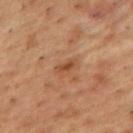No biopsy was performed on this lesion — it was imaged during a full skin examination and was not determined to be concerning.
This is a cross-polarized tile.
A male subject approximately 55 years of age.
A roughly 15 mm field-of-view crop from a total-body skin photograph.
Located on the upper back.
Approximately 2.5 mm at its widest.